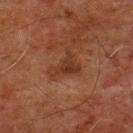Clinical impression:
Captured during whole-body skin photography for melanoma surveillance; the lesion was not biopsied.
Background:
This is a cross-polarized tile. The subject is a male roughly 80 years of age. Cropped from a total-body skin-imaging series; the visible field is about 15 mm. Longest diameter approximately 3.5 mm. An algorithmic analysis of the crop reported a color-variation rating of about 2.5/10 and peripheral color asymmetry of about 1. On the upper back.